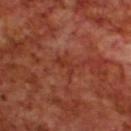notes: total-body-photography surveillance lesion; no biopsy